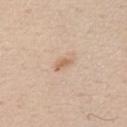The total-body-photography lesion software estimated an area of roughly 2.5 mm², a shape eccentricity near 0.85, and two-axis asymmetry of about 0.25. The software also gave a border-irregularity rating of about 2.5/10, internal color variation of about 0.5 on a 0–10 scale, and peripheral color asymmetry of about 0. The analysis additionally found an automated nevus-likeness rating near 15 out of 100 and a lesion-detection confidence of about 100/100. About 2.5 mm across. This is a white-light tile. A lesion tile, about 15 mm wide, cut from a 3D total-body photograph. The lesion is on the chest. A male subject aged 58–62.